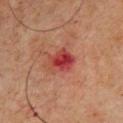Q: Was a biopsy performed?
A: total-body-photography surveillance lesion; no biopsy
Q: Lesion size?
A: ≈3.5 mm
Q: What is the imaging modality?
A: 15 mm crop, total-body photography
Q: Lesion location?
A: the front of the torso
Q: What did automated image analysis measure?
A: an area of roughly 7 mm², an eccentricity of roughly 0.6, and a shape-asymmetry score of about 0.25 (0 = symmetric)
Q: Who is the patient?
A: male, aged around 65